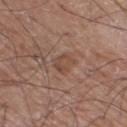Cropped from a whole-body photographic skin survey; the tile spans about 15 mm.
The total-body-photography lesion software estimated an area of roughly 4.5 mm², an eccentricity of roughly 0.75, and a symmetry-axis asymmetry near 0.25. The analysis additionally found a border-irregularity index near 3/10, a color-variation rating of about 2.5/10, and peripheral color asymmetry of about 1. And it measured a detector confidence of about 100 out of 100 that the crop contains a lesion.
On the right thigh.
A male patient, aged 58 to 62.
Measured at roughly 2.5 mm in maximum diameter.
This is a white-light tile.